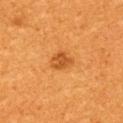Clinical impression: This lesion was catalogued during total-body skin photography and was not selected for biopsy. Clinical summary: On the upper back. The total-body-photography lesion software estimated a lesion area of about 5 mm², an eccentricity of roughly 0.6, and a symmetry-axis asymmetry near 0.25. A lesion tile, about 15 mm wide, cut from a 3D total-body photograph. A male patient about 60 years old. This is a cross-polarized tile. The recorded lesion diameter is about 2.5 mm.